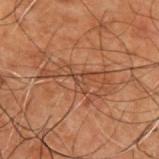This is a cross-polarized tile. Approximately 6.5 mm at its widest. A 15 mm close-up extracted from a 3D total-body photography capture. Located on the upper back. A male subject, roughly 50 years of age.